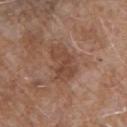Q: How was the tile lit?
A: white-light
Q: What is the anatomic site?
A: the upper back
Q: What is the imaging modality?
A: ~15 mm crop, total-body skin-cancer survey
Q: What are the patient's age and sex?
A: male, aged approximately 60